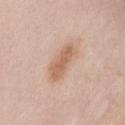• workup: catalogued during a skin exam; not biopsied
• body site: the chest
• subject: female, roughly 45 years of age
• image: total-body-photography crop, ~15 mm field of view